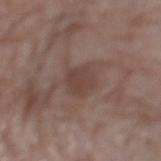Recorded during total-body skin imaging; not selected for excision or biopsy.
A female subject, approximately 70 years of age.
Automated tile analysis of the lesion measured a lesion area of about 7.5 mm², an outline eccentricity of about 0.75 (0 = round, 1 = elongated), and a symmetry-axis asymmetry near 0.25. The software also gave a nevus-likeness score of about 0/100.
On the left lower leg.
A 15 mm close-up tile from a total-body photography series done for melanoma screening.
The tile uses white-light illumination.
Measured at roughly 4 mm in maximum diameter.